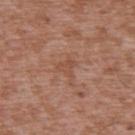workup — catalogued during a skin exam; not biopsied
patient — male, about 45 years old
location — the upper back
acquisition — ~15 mm tile from a whole-body skin photo